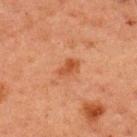biopsy_status: not biopsied; imaged during a skin examination
lighting: cross-polarized
image:
  source: total-body photography crop
  field_of_view_mm: 15
lesion_size:
  long_diameter_mm_approx: 3.0
patient:
  sex: male
  age_approx: 60
automated_metrics:
  area_mm2_approx: 4.0
  eccentricity: 0.8
  cielab_L: 41
  cielab_a: 24
  cielab_b: 32
  vs_skin_contrast_norm: 7.0
  border_irregularity_0_10: 2.5
  color_variation_0_10: 2.5
site: upper back